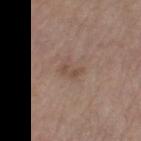follow-up — total-body-photography surveillance lesion; no biopsy
subject — female, aged 63–67
lighting — white-light
body site — the left thigh
size — ~2.5 mm (longest diameter)
automated metrics — an average lesion color of about L≈49 a*≈17 b*≈25 (CIELAB), about 7 CIELAB-L* units darker than the surrounding skin, and a normalized lesion–skin contrast near 5.5; a classifier nevus-likeness of about 0/100
acquisition — ~15 mm tile from a whole-body skin photo